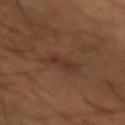Captured during whole-body skin photography for melanoma surveillance; the lesion was not biopsied. The lesion-visualizer software estimated an average lesion color of about L≈32 a*≈20 b*≈26 (CIELAB) and a lesion-to-skin contrast of about 6 (normalized; higher = more distinct). The analysis additionally found border irregularity of about 3.5 on a 0–10 scale, a within-lesion color-variation index near 0.5/10, and radial color variation of about 0. And it measured an automated nevus-likeness rating near 0 out of 100. From the left forearm. Imaged with cross-polarized lighting. A male patient, about 50 years old. Cropped from a whole-body photographic skin survey; the tile spans about 15 mm.